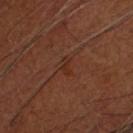Q: Is there a histopathology result?
A: no biopsy performed (imaged during a skin exam)
Q: Illumination type?
A: cross-polarized illumination
Q: What are the patient's age and sex?
A: male, about 65 years old
Q: Automated lesion metrics?
A: a lesion color around L≈27 a*≈22 b*≈27 in CIELAB, roughly 5 lightness units darker than nearby skin, and a normalized lesion–skin contrast near 5.5; border irregularity of about 5 on a 0–10 scale and a peripheral color-asymmetry measure near 0
Q: How large is the lesion?
A: ≈2 mm
Q: What is the imaging modality?
A: ~15 mm crop, total-body skin-cancer survey
Q: Where on the body is the lesion?
A: the head or neck